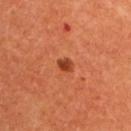body site=the back
image=~15 mm tile from a whole-body skin photo
patient=female, in their mid-60s
automated metrics=a footprint of about 2.5 mm² and an eccentricity of roughly 0.65; an average lesion color of about L≈42 a*≈33 b*≈39 (CIELAB), about 12 CIELAB-L* units darker than the surrounding skin, and a normalized lesion–skin contrast near 9; a border-irregularity index near 1/10, a within-lesion color-variation index near 2/10, and a peripheral color-asymmetry measure near 0.5; lesion-presence confidence of about 100/100
lesion size=about 2 mm
tile lighting=cross-polarized